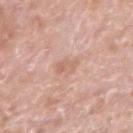The lesion was tiled from a total-body skin photograph and was not biopsied.
An algorithmic analysis of the crop reported a lesion area of about 4 mm², an eccentricity of roughly 0.75, and a symmetry-axis asymmetry near 0.35. The software also gave a lesion color around L≈63 a*≈21 b*≈29 in CIELAB and roughly 7 lightness units darker than nearby skin. The analysis additionally found a classifier nevus-likeness of about 0/100.
On the arm.
The tile uses white-light illumination.
Longest diameter approximately 2.5 mm.
The subject is a male about 50 years old.
A roughly 15 mm field-of-view crop from a total-body skin photograph.